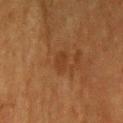The lesion was photographed on a routine skin check and not biopsied; there is no pathology result.